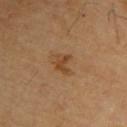Impression:
Part of a total-body skin-imaging series; this lesion was reviewed on a skin check and was not flagged for biopsy.
Image and clinical context:
A lesion tile, about 15 mm wide, cut from a 3D total-body photograph. A male subject, approximately 70 years of age. The lesion is on the back.